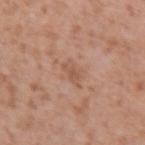Imaged during a routine full-body skin examination; the lesion was not biopsied and no histopathology is available. Automated tile analysis of the lesion measured roughly 7 lightness units darker than nearby skin and a normalized lesion–skin contrast near 5.5. It also reported an automated nevus-likeness rating near 0 out of 100 and a lesion-detection confidence of about 100/100. Cropped from a total-body skin-imaging series; the visible field is about 15 mm. The recorded lesion diameter is about 3 mm. A female subject aged 38 to 42. The lesion is on the arm.